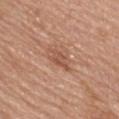Impression: The lesion was tiled from a total-body skin photograph and was not biopsied. Background: A 15 mm close-up tile from a total-body photography series done for melanoma screening. A female subject, approximately 65 years of age. Located on the upper back.